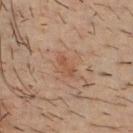Case summary:
* biopsy status: imaged on a skin check; not biopsied
* location: the chest
* lighting: cross-polarized illumination
* patient: male, about 35 years old
* image source: total-body-photography crop, ~15 mm field of view
* lesion diameter: about 3 mm
* automated metrics: a footprint of about 3.5 mm² and an outline eccentricity of about 0.9 (0 = round, 1 = elongated); an average lesion color of about L≈46 a*≈19 b*≈29 (CIELAB), about 6 CIELAB-L* units darker than the surrounding skin, and a lesion-to-skin contrast of about 5.5 (normalized; higher = more distinct); a nevus-likeness score of about 0/100 and a detector confidence of about 100 out of 100 that the crop contains a lesion